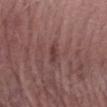Impression:
Recorded during total-body skin imaging; not selected for excision or biopsy.
Clinical summary:
This image is a 15 mm lesion crop taken from a total-body photograph. A male subject about 65 years old. The tile uses white-light illumination. From the arm.